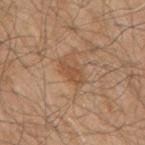Q: Is there a histopathology result?
A: no biopsy performed (imaged during a skin exam)
Q: Who is the patient?
A: male, aged 78 to 82
Q: What kind of image is this?
A: 15 mm crop, total-body photography
Q: Illumination type?
A: white-light illumination
Q: What is the anatomic site?
A: the right upper arm
Q: What is the lesion's diameter?
A: ≈3.5 mm